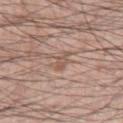follow-up = imaged on a skin check; not biopsied | imaging modality = ~15 mm crop, total-body skin-cancer survey | lesion diameter = ~3 mm (longest diameter) | subject = male, aged 58 to 62 | location = the right thigh | image-analysis metrics = a within-lesion color-variation index near 0/10; a nevus-likeness score of about 0/100 and lesion-presence confidence of about 95/100 | lighting = white-light illumination.